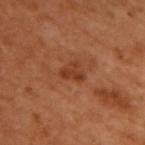follow-up — imaged on a skin check; not biopsied
acquisition — total-body-photography crop, ~15 mm field of view
site — the upper back
subject — male, about 50 years old
lighting — cross-polarized illumination
lesion size — about 2.5 mm